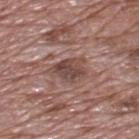Captured during whole-body skin photography for melanoma surveillance; the lesion was not biopsied.
A 15 mm close-up extracted from a 3D total-body photography capture.
The subject is a male approximately 70 years of age.
Located on the upper back.